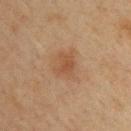Q: Is there a histopathology result?
A: total-body-photography surveillance lesion; no biopsy
Q: What kind of image is this?
A: ~15 mm crop, total-body skin-cancer survey
Q: What lighting was used for the tile?
A: cross-polarized illumination
Q: Patient demographics?
A: male, in their 40s
Q: What is the anatomic site?
A: the upper back